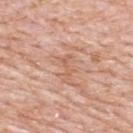Imaged during a routine full-body skin examination; the lesion was not biopsied and no histopathology is available. Imaged with white-light lighting. Measured at roughly 3 mm in maximum diameter. The lesion is located on the upper back. Automated image analysis of the tile measured a footprint of about 3 mm², an eccentricity of roughly 0.9, and a shape-asymmetry score of about 0.5 (0 = symmetric). And it measured a nevus-likeness score of about 0/100 and a detector confidence of about 65 out of 100 that the crop contains a lesion. A lesion tile, about 15 mm wide, cut from a 3D total-body photograph. A male patient, aged 78 to 82.